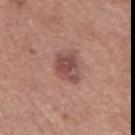Clinical impression:
Part of a total-body skin-imaging series; this lesion was reviewed on a skin check and was not flagged for biopsy.
Background:
The total-body-photography lesion software estimated a border-irregularity rating of about 2/10, internal color variation of about 4 on a 0–10 scale, and a peripheral color-asymmetry measure near 1.5. The analysis additionally found an automated nevus-likeness rating near 40 out of 100. A 15 mm close-up tile from a total-body photography series done for melanoma screening. A male patient, about 75 years old. This is a white-light tile. The lesion is located on the mid back. The recorded lesion diameter is about 3.5 mm.